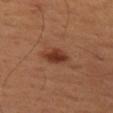| field | value |
|---|---|
| workup | catalogued during a skin exam; not biopsied |
| anatomic site | the left thigh |
| tile lighting | cross-polarized illumination |
| acquisition | ~15 mm tile from a whole-body skin photo |
| diameter | ≈3.5 mm |
| subject | male, aged approximately 50 |
| automated metrics | a border-irregularity rating of about 1.5/10 and a color-variation rating of about 3/10; an automated nevus-likeness rating near 95 out of 100 and a detector confidence of about 100 out of 100 that the crop contains a lesion |